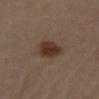Q: Was a biopsy performed?
A: catalogued during a skin exam; not biopsied
Q: Automated lesion metrics?
A: a lesion color around L≈34 a*≈16 b*≈25 in CIELAB, about 10 CIELAB-L* units darker than the surrounding skin, and a normalized border contrast of about 9.5
Q: Patient demographics?
A: female, aged around 65
Q: How large is the lesion?
A: about 4 mm
Q: How was the tile lit?
A: cross-polarized
Q: How was this image acquired?
A: total-body-photography crop, ~15 mm field of view
Q: Lesion location?
A: the right thigh